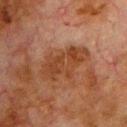Background: Imaged with cross-polarized lighting. The lesion's longest dimension is about 6 mm. A male subject, approximately 80 years of age. This image is a 15 mm lesion crop taken from a total-body photograph. An algorithmic analysis of the crop reported an area of roughly 15 mm². The analysis additionally found a lesion color around L≈33 a*≈20 b*≈28 in CIELAB, a lesion–skin lightness drop of about 7, and a normalized border contrast of about 7.5. It also reported a border-irregularity index near 4/10, internal color variation of about 3 on a 0–10 scale, and a peripheral color-asymmetry measure near 1. The analysis additionally found an automated nevus-likeness rating near 0 out of 100 and lesion-presence confidence of about 100/100. From the chest.